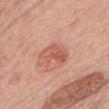Q: Was this lesion biopsied?
A: imaged on a skin check; not biopsied
Q: What lighting was used for the tile?
A: white-light
Q: Who is the patient?
A: female, in their 60s
Q: How large is the lesion?
A: about 4 mm
Q: Lesion location?
A: the head or neck
Q: What kind of image is this?
A: ~15 mm crop, total-body skin-cancer survey
Q: Automated lesion metrics?
A: internal color variation of about 4 on a 0–10 scale and peripheral color asymmetry of about 1.5